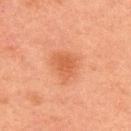Impression: Imaged during a routine full-body skin examination; the lesion was not biopsied and no histopathology is available. Background: A male subject approximately 50 years of age. Approximately 4 mm at its widest. Automated image analysis of the tile measured a within-lesion color-variation index near 2/10 and peripheral color asymmetry of about 0.5. The software also gave lesion-presence confidence of about 100/100. A 15 mm close-up tile from a total-body photography series done for melanoma screening. The lesion is on the upper back. Captured under cross-polarized illumination.